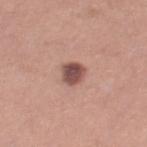Q: Was this lesion biopsied?
A: total-body-photography surveillance lesion; no biopsy
Q: Who is the patient?
A: female, aged approximately 30
Q: Where on the body is the lesion?
A: the right thigh
Q: What did automated image analysis measure?
A: a classifier nevus-likeness of about 65/100
Q: Lesion size?
A: about 3 mm
Q: Illumination type?
A: white-light illumination
Q: How was this image acquired?
A: ~15 mm tile from a whole-body skin photo